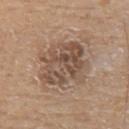Part of a total-body skin-imaging series; this lesion was reviewed on a skin check and was not flagged for biopsy.
The lesion is located on the left upper arm.
The subject is a male aged approximately 80.
This image is a 15 mm lesion crop taken from a total-body photograph.